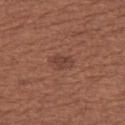Case summary:
* follow-up — catalogued during a skin exam; not biopsied
* body site — the left thigh
* diameter — ≈2.5 mm
* automated lesion analysis — an average lesion color of about L≈41 a*≈22 b*≈25 (CIELAB) and a lesion-to-skin contrast of about 6.5 (normalized; higher = more distinct); a border-irregularity index near 3/10
* image — ~15 mm crop, total-body skin-cancer survey
* subject — female, aged approximately 55
* tile lighting — white-light illumination